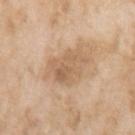Image and clinical context:
The recorded lesion diameter is about 6 mm. Imaged with white-light lighting. From the left upper arm. A lesion tile, about 15 mm wide, cut from a 3D total-body photograph. A female subject, in their mid- to late 70s.